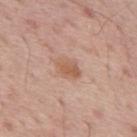biopsy status: total-body-photography surveillance lesion; no biopsy | automated metrics: a shape-asymmetry score of about 0.2 (0 = symmetric); about 8 CIELAB-L* units darker than the surrounding skin and a lesion-to-skin contrast of about 6.5 (normalized; higher = more distinct) | image source: ~15 mm tile from a whole-body skin photo | size: ≈3.5 mm | subject: male, aged around 65 | site: the back | lighting: white-light illumination.